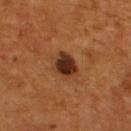biopsy status = imaged on a skin check; not biopsied.